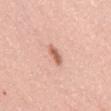Background:
A lesion tile, about 15 mm wide, cut from a 3D total-body photograph. From the mid back. The patient is a male aged around 60.